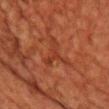notes = total-body-photography surveillance lesion; no biopsy
automated metrics = a border-irregularity index near 9/10, a within-lesion color-variation index near 1/10, and radial color variation of about 0; a nevus-likeness score of about 0/100
diameter = about 4.5 mm
site = the chest
image = ~15 mm tile from a whole-body skin photo
subject = male, in their 60s